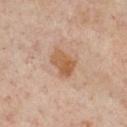A lesion tile, about 15 mm wide, cut from a 3D total-body photograph. Located on the chest. Longest diameter approximately 4 mm. This is a cross-polarized tile. A patient aged 53 to 57.